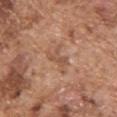| field | value |
|---|---|
| notes | no biopsy performed (imaged during a skin exam) |
| imaging modality | total-body-photography crop, ~15 mm field of view |
| image-analysis metrics | border irregularity of about 6.5 on a 0–10 scale, a within-lesion color-variation index near 2.5/10, and peripheral color asymmetry of about 1; a detector confidence of about 80 out of 100 that the crop contains a lesion |
| lighting | white-light illumination |
| site | the front of the torso |
| patient | male, about 75 years old |